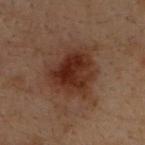biopsy status: imaged on a skin check; not biopsied | patient: male, about 30 years old | diameter: about 5.5 mm | tile lighting: cross-polarized | image source: ~15 mm tile from a whole-body skin photo | anatomic site: the upper back.